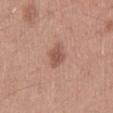This lesion was catalogued during total-body skin photography and was not selected for biopsy.
A male subject, aged 28–32.
The tile uses white-light illumination.
The total-body-photography lesion software estimated a lesion color around L≈53 a*≈22 b*≈26 in CIELAB and a lesion-to-skin contrast of about 7 (normalized; higher = more distinct). It also reported a color-variation rating of about 2.5/10 and a peripheral color-asymmetry measure near 0.5.
A 15 mm close-up extracted from a 3D total-body photography capture.
The lesion is located on the mid back.
Longest diameter approximately 3.5 mm.